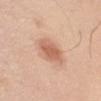Approximately 3.5 mm at its widest.
The patient is a male in their mid- to late 70s.
A 15 mm crop from a total-body photograph taken for skin-cancer surveillance.
The tile uses white-light illumination.
Located on the left upper arm.